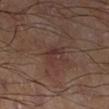Imaged during a routine full-body skin examination; the lesion was not biopsied and no histopathology is available.
Located on the leg.
The total-body-photography lesion software estimated a footprint of about 5.5 mm², a shape eccentricity near 0.75, and a shape-asymmetry score of about 0.25 (0 = symmetric). The software also gave a lesion–skin lightness drop of about 6 and a lesion-to-skin contrast of about 6 (normalized; higher = more distinct).
A 15 mm close-up tile from a total-body photography series done for melanoma screening.
Longest diameter approximately 3.5 mm.
This is a cross-polarized tile.